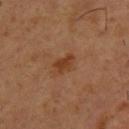Assessment:
No biopsy was performed on this lesion — it was imaged during a full skin examination and was not determined to be concerning.
Background:
A male subject, aged 53 to 57. Automated image analysis of the tile measured an average lesion color of about L≈38 a*≈22 b*≈33 (CIELAB), a lesion–skin lightness drop of about 8, and a normalized border contrast of about 8. It also reported a nevus-likeness score of about 70/100 and a lesion-detection confidence of about 100/100. The recorded lesion diameter is about 2.5 mm. A region of skin cropped from a whole-body photographic capture, roughly 15 mm wide. On the upper back.